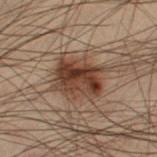This lesion was catalogued during total-body skin photography and was not selected for biopsy. A male patient roughly 55 years of age. The lesion is on the right thigh. A 15 mm crop from a total-body photograph taken for skin-cancer surveillance. Longest diameter approximately 5 mm.